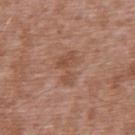Impression:
The lesion was photographed on a routine skin check and not biopsied; there is no pathology result.
Acquisition and patient details:
A male patient, roughly 45 years of age. This is a white-light tile. A roughly 15 mm field-of-view crop from a total-body skin photograph. On the upper back.